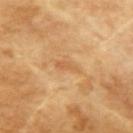lighting: cross-polarized | lesion size: about 2.5 mm | TBP lesion metrics: a lesion area of about 2.5 mm² and two-axis asymmetry of about 0.3; a mean CIELAB color near L≈60 a*≈22 b*≈42, roughly 7 lightness units darker than nearby skin, and a normalized lesion–skin contrast near 5; border irregularity of about 3.5 on a 0–10 scale, internal color variation of about 0 on a 0–10 scale, and a peripheral color-asymmetry measure near 0; a classifier nevus-likeness of about 0/100 and lesion-presence confidence of about 100/100 | imaging modality: total-body-photography crop, ~15 mm field of view | patient: male, aged approximately 65 | site: the left upper arm.